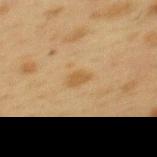workup: catalogued during a skin exam; not biopsied
patient: female, aged 38 to 42
location: the upper back
acquisition: 15 mm crop, total-body photography